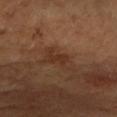notes: imaged on a skin check; not biopsied
image source: ~15 mm crop, total-body skin-cancer survey
location: the left forearm
size: ~2.5 mm (longest diameter)
illumination: cross-polarized
image-analysis metrics: a nevus-likeness score of about 0/100 and lesion-presence confidence of about 100/100
patient: female, in their mid- to late 50s